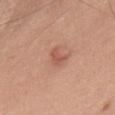{"biopsy_status": "not biopsied; imaged during a skin examination", "image": {"source": "total-body photography crop", "field_of_view_mm": 15}, "patient": {"sex": "male", "age_approx": 75}, "site": "upper back"}